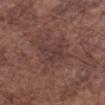This lesion was catalogued during total-body skin photography and was not selected for biopsy.
A male subject, about 75 years old.
This is a white-light tile.
Located on the right forearm.
An algorithmic analysis of the crop reported a lesion-detection confidence of about 60/100.
A close-up tile cropped from a whole-body skin photograph, about 15 mm across.
Measured at roughly 3.5 mm in maximum diameter.